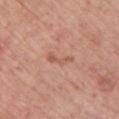{
  "biopsy_status": "not biopsied; imaged during a skin examination",
  "lighting": "white-light",
  "site": "chest",
  "automated_metrics": {
    "area_mm2_approx": 3.5,
    "eccentricity": 0.95,
    "shape_asymmetry": 0.55,
    "cielab_L": 57,
    "cielab_a": 23,
    "cielab_b": 30,
    "vs_skin_darker_L": 7.0,
    "vs_skin_contrast_norm": 5.5,
    "nevus_likeness_0_100": 0,
    "lesion_detection_confidence_0_100": 100
  },
  "patient": {
    "sex": "male",
    "age_approx": 60
  },
  "image": {
    "source": "total-body photography crop",
    "field_of_view_mm": 15
  }
}